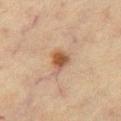{"biopsy_status": "not biopsied; imaged during a skin examination", "site": "leg", "image": {"source": "total-body photography crop", "field_of_view_mm": 15}, "automated_metrics": {"cielab_L": 47, "cielab_a": 18, "cielab_b": 30, "vs_skin_darker_L": 11.0, "border_irregularity_0_10": 3.5, "color_variation_0_10": 4.0, "peripheral_color_asymmetry": 1.0}, "patient": {"sex": "female", "age_approx": 65}, "lighting": "cross-polarized"}